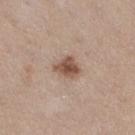Q: Who is the patient?
A: female, aged 38 to 42
Q: What did automated image analysis measure?
A: border irregularity of about 3 on a 0–10 scale and peripheral color asymmetry of about 1; an automated nevus-likeness rating near 95 out of 100 and a lesion-detection confidence of about 100/100
Q: How was the tile lit?
A: white-light illumination
Q: What is the imaging modality?
A: ~15 mm crop, total-body skin-cancer survey
Q: Lesion size?
A: ~3 mm (longest diameter)
Q: What is the anatomic site?
A: the leg